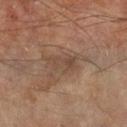Imaged during a routine full-body skin examination; the lesion was not biopsied and no histopathology is available. This image is a 15 mm lesion crop taken from a total-body photograph. The lesion is on the left forearm. The patient is a male about 70 years old. Imaged with cross-polarized lighting. The lesion-visualizer software estimated a lesion area of about 5.5 mm² and a shape eccentricity near 0.8. It also reported a mean CIELAB color near L≈44 a*≈16 b*≈26 and about 6 CIELAB-L* units darker than the surrounding skin. The software also gave a lesion-detection confidence of about 95/100.